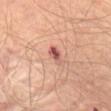This lesion was catalogued during total-body skin photography and was not selected for biopsy.
The tile uses cross-polarized illumination.
Automated image analysis of the tile measured an outline eccentricity of about 0.8 (0 = round, 1 = elongated) and a symmetry-axis asymmetry near 0.2. And it measured an average lesion color of about L≈55 a*≈26 b*≈26 (CIELAB) and a lesion–skin lightness drop of about 12. And it measured an automated nevus-likeness rating near 15 out of 100 and a detector confidence of about 100 out of 100 that the crop contains a lesion.
The patient is a male aged around 65.
From the abdomen.
Cropped from a whole-body photographic skin survey; the tile spans about 15 mm.
Approximately 2.5 mm at its widest.